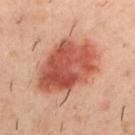biopsy status — catalogued during a skin exam; not biopsied | location — the upper back | patient — male, approximately 40 years of age | imaging modality — 15 mm crop, total-body photography | illumination — cross-polarized illumination | diameter — about 8 mm | TBP lesion metrics — a lesion color around L≈53 a*≈28 b*≈32 in CIELAB, a lesion–skin lightness drop of about 14, and a lesion-to-skin contrast of about 9.5 (normalized; higher = more distinct); a border-irregularity rating of about 2/10, a within-lesion color-variation index near 7/10, and a peripheral color-asymmetry measure near 2.5.